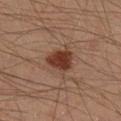About 3.5 mm across. A roughly 15 mm field-of-view crop from a total-body skin photograph. The tile uses cross-polarized illumination. On the right lower leg. The patient is a male in their 40s.